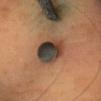Captured during whole-body skin photography for melanoma surveillance; the lesion was not biopsied.
The lesion is on the head or neck.
The recorded lesion diameter is about 4.5 mm.
A 15 mm close-up tile from a total-body photography series done for melanoma screening.
A female patient roughly 50 years of age.
Imaged with cross-polarized lighting.